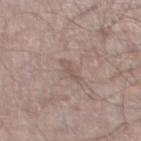A roughly 15 mm field-of-view crop from a total-body skin photograph. This is a white-light tile. From the left thigh. An algorithmic analysis of the crop reported border irregularity of about 4.5 on a 0–10 scale, internal color variation of about 0 on a 0–10 scale, and radial color variation of about 0. It also reported an automated nevus-likeness rating near 0 out of 100 and a lesion-detection confidence of about 100/100. Approximately 2.5 mm at its widest. A male patient in their mid-50s.